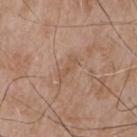Clinical impression: Recorded during total-body skin imaging; not selected for excision or biopsy. Image and clinical context: The lesion is on the chest. Cropped from a total-body skin-imaging series; the visible field is about 15 mm. A male patient aged approximately 65.